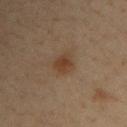Assessment:
Captured during whole-body skin photography for melanoma surveillance; the lesion was not biopsied.
Context:
Cropped from a whole-body photographic skin survey; the tile spans about 15 mm. On the right upper arm. The subject is a male in their mid- to late 30s. Imaged with cross-polarized lighting. The recorded lesion diameter is about 2.5 mm.